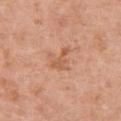Assessment: Recorded during total-body skin imaging; not selected for excision or biopsy. Clinical summary: The tile uses white-light illumination. A 15 mm close-up tile from a total-body photography series done for melanoma screening. The lesion's longest dimension is about 3 mm. The lesion-visualizer software estimated an area of roughly 4 mm², a shape eccentricity near 0.9, and two-axis asymmetry of about 0.5. The patient is a female aged around 50. On the chest.